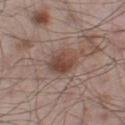– workup: total-body-photography surveillance lesion; no biopsy
– image: total-body-photography crop, ~15 mm field of view
– body site: the left thigh
– patient: male, approximately 55 years of age